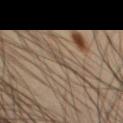{
  "biopsy_status": "not biopsied; imaged during a skin examination",
  "site": "chest",
  "image": {
    "source": "total-body photography crop",
    "field_of_view_mm": 15
  },
  "patient": {
    "sex": "male",
    "age_approx": 35
  },
  "lighting": "cross-polarized"
}